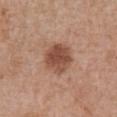Assessment: Part of a total-body skin-imaging series; this lesion was reviewed on a skin check and was not flagged for biopsy. Clinical summary: Longest diameter approximately 3.5 mm. Automated tile analysis of the lesion measured a lesion area of about 10 mm², an outline eccentricity of about 0.35 (0 = round, 1 = elongated), and a symmetry-axis asymmetry near 0.15. The analysis additionally found a border-irregularity rating of about 1.5/10, a color-variation rating of about 3.5/10, and a peripheral color-asymmetry measure near 1. The analysis additionally found a lesion-detection confidence of about 100/100. The lesion is located on the front of the torso. A region of skin cropped from a whole-body photographic capture, roughly 15 mm wide. A male subject, aged 68 to 72.